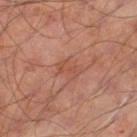workup=catalogued during a skin exam; not biopsied | image=~15 mm crop, total-body skin-cancer survey | body site=the left thigh | patient=male, aged around 55.